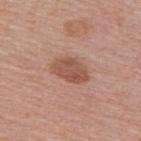biopsy_status: not biopsied; imaged during a skin examination
site: upper back
lighting: white-light
patient:
  sex: female
  age_approx: 60
image:
  source: total-body photography crop
  field_of_view_mm: 15